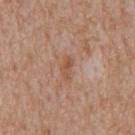Q: Is there a histopathology result?
A: no biopsy performed (imaged during a skin exam)
Q: What is the imaging modality?
A: ~15 mm tile from a whole-body skin photo
Q: Patient demographics?
A: male, aged 63 to 67
Q: Lesion location?
A: the mid back